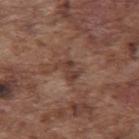{
  "automated_metrics": {
    "area_mm2_approx": 4.0,
    "shape_asymmetry": 0.45,
    "cielab_L": 39,
    "cielab_a": 19,
    "cielab_b": 24,
    "vs_skin_darker_L": 8.0,
    "vs_skin_contrast_norm": 7.0
  },
  "site": "upper back",
  "image": {
    "source": "total-body photography crop",
    "field_of_view_mm": 15
  },
  "patient": {
    "sex": "male",
    "age_approx": 75
  },
  "lighting": "white-light",
  "lesion_size": {
    "long_diameter_mm_approx": 3.0
  }
}